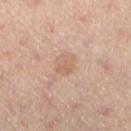Acquisition and patient details: A female patient aged 43 to 47. Measured at roughly 2.5 mm in maximum diameter. A 15 mm close-up tile from a total-body photography series done for melanoma screening. On the left lower leg. This is a cross-polarized tile. An algorithmic analysis of the crop reported a footprint of about 5 mm² and a shape-asymmetry score of about 0.2 (0 = symmetric). The software also gave a border-irregularity index near 2/10, internal color variation of about 2 on a 0–10 scale, and a peripheral color-asymmetry measure near 0.5.